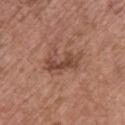biopsy status = catalogued during a skin exam; not biopsied
lesion diameter = about 5 mm
illumination = white-light
image source = ~15 mm tile from a whole-body skin photo
TBP lesion metrics = border irregularity of about 6.5 on a 0–10 scale; a classifier nevus-likeness of about 5/100 and a detector confidence of about 100 out of 100 that the crop contains a lesion
location = the upper back
patient = female, roughly 65 years of age